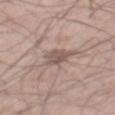{
  "biopsy_status": "not biopsied; imaged during a skin examination",
  "patient": {
    "sex": "male",
    "age_approx": 55
  },
  "lighting": "white-light",
  "site": "mid back",
  "image": {
    "source": "total-body photography crop",
    "field_of_view_mm": 15
  },
  "automated_metrics": {
    "area_mm2_approx": 5.5,
    "eccentricity": 0.6,
    "shape_asymmetry": 0.25,
    "border_irregularity_0_10": 3.0,
    "peripheral_color_asymmetry": 1.0,
    "nevus_likeness_0_100": 0,
    "lesion_detection_confidence_0_100": 80
  },
  "lesion_size": {
    "long_diameter_mm_approx": 3.0
  }
}